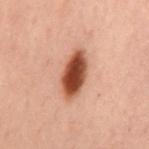size: ≈5.5 mm
patient: female, about 55 years old
body site: the mid back
tile lighting: cross-polarized
acquisition: ~15 mm tile from a whole-body skin photo